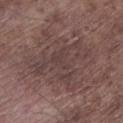Clinical impression:
Captured during whole-body skin photography for melanoma surveillance; the lesion was not biopsied.
Acquisition and patient details:
Automated image analysis of the tile measured an area of roughly 27 mm². And it measured a border-irregularity index near 7/10. A male subject, in their mid- to late 70s. On the left lower leg. The lesion's longest dimension is about 7.5 mm. The tile uses white-light illumination. A 15 mm close-up tile from a total-body photography series done for melanoma screening.